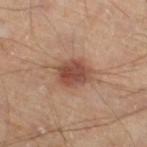Part of a total-body skin-imaging series; this lesion was reviewed on a skin check and was not flagged for biopsy. From the leg. The patient is a male aged 43 to 47. A region of skin cropped from a whole-body photographic capture, roughly 15 mm wide. The tile uses cross-polarized illumination.